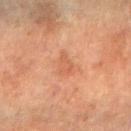notes = no biopsy performed (imaged during a skin exam) | image = 15 mm crop, total-body photography | illumination = cross-polarized | subject = female, approximately 55 years of age | anatomic site = the right forearm | lesion size = ~3 mm (longest diameter).